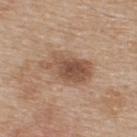Case summary:
– workup — imaged on a skin check; not biopsied
– lesion diameter — about 5.5 mm
– image — ~15 mm crop, total-body skin-cancer survey
– tile lighting — white-light illumination
– patient — male, in their mid-70s
– TBP lesion metrics — border irregularity of about 3.5 on a 0–10 scale and radial color variation of about 2
– anatomic site — the upper back